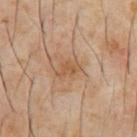The lesion was tiled from a total-body skin photograph and was not biopsied. Longest diameter approximately 3 mm. The tile uses cross-polarized illumination. A male patient, in their 60s. A roughly 15 mm field-of-view crop from a total-body skin photograph. From the abdomen. An algorithmic analysis of the crop reported a mean CIELAB color near L≈50 a*≈18 b*≈32 and a lesion-to-skin contrast of about 6 (normalized; higher = more distinct).